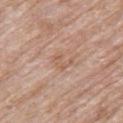The lesion was tiled from a total-body skin photograph and was not biopsied.
A close-up tile cropped from a whole-body skin photograph, about 15 mm across.
This is a white-light tile.
A female patient, aged approximately 85 or older.
On the upper back.
An algorithmic analysis of the crop reported an average lesion color of about L≈58 a*≈20 b*≈30 (CIELAB), a lesion–skin lightness drop of about 6, and a lesion-to-skin contrast of about 5 (normalized; higher = more distinct).
The lesion's longest dimension is about 2.5 mm.